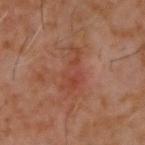Image and clinical context: On the upper back. This image is a 15 mm lesion crop taken from a total-body photograph. Automated tile analysis of the lesion measured about 6 CIELAB-L* units darker than the surrounding skin and a normalized border contrast of about 5.5. It also reported border irregularity of about 7 on a 0–10 scale and radial color variation of about 1. And it measured a classifier nevus-likeness of about 0/100 and a lesion-detection confidence of about 100/100. The tile uses cross-polarized illumination. A male subject approximately 60 years of age.